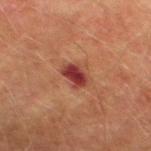<tbp_lesion>
  <biopsy_status>not biopsied; imaged during a skin examination</biopsy_status>
  <patient>
    <sex>male</sex>
    <age_approx>75</age_approx>
  </patient>
  <site>left thigh</site>
  <image>
    <source>total-body photography crop</source>
    <field_of_view_mm>15</field_of_view_mm>
  </image>
</tbp_lesion>